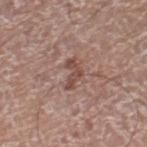Captured during whole-body skin photography for melanoma surveillance; the lesion was not biopsied.
Located on the right lower leg.
The recorded lesion diameter is about 3.5 mm.
A male subject aged 73–77.
The total-body-photography lesion software estimated a lesion color around L≈48 a*≈20 b*≈25 in CIELAB, roughly 9 lightness units darker than nearby skin, and a normalized border contrast of about 7.
Imaged with white-light lighting.
A 15 mm close-up extracted from a 3D total-body photography capture.